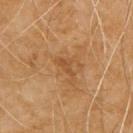Captured during whole-body skin photography for melanoma surveillance; the lesion was not biopsied. Captured under cross-polarized illumination. A male patient approximately 60 years of age. The lesion is on the back. Cropped from a total-body skin-imaging series; the visible field is about 15 mm. The total-body-photography lesion software estimated an area of roughly 2 mm² and a symmetry-axis asymmetry near 0.4. And it measured a nevus-likeness score of about 0/100 and a lesion-detection confidence of about 100/100.